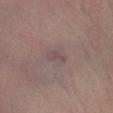The lesion was tiled from a total-body skin photograph and was not biopsied.
A male patient, aged 58 to 62.
Automated image analysis of the tile measured about 6 CIELAB-L* units darker than the surrounding skin and a normalized border contrast of about 5.5. And it measured a color-variation rating of about 1/10 and peripheral color asymmetry of about 0.
Measured at roughly 2.5 mm in maximum diameter.
A 15 mm close-up tile from a total-body photography series done for melanoma screening.
The tile uses white-light illumination.
From the left lower leg.